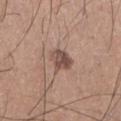follow-up: total-body-photography surveillance lesion; no biopsy | subject: male, approximately 30 years of age | body site: the left lower leg | imaging modality: ~15 mm crop, total-body skin-cancer survey | lesion diameter: ~3 mm (longest diameter).